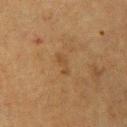workup: no biopsy performed (imaged during a skin exam) | image source: ~15 mm crop, total-body skin-cancer survey | site: the left upper arm | size: ~2.5 mm (longest diameter) | patient: female, in their mid- to late 50s | lighting: cross-polarized illumination.